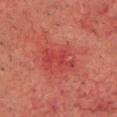biopsy_status: not biopsied; imaged during a skin examination
lesion_size:
  long_diameter_mm_approx: 4.0
automated_metrics:
  area_mm2_approx: 5.5
  eccentricity: 0.9
  nevus_likeness_0_100: 5
  lesion_detection_confidence_0_100: 100
patient:
  sex: male
  age_approx: 75
lighting: cross-polarized
site: head or neck
image:
  source: total-body photography crop
  field_of_view_mm: 15